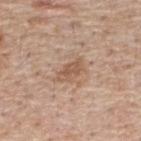Case summary:
– workup · catalogued during a skin exam; not biopsied
– patient · male, aged around 55
– image-analysis metrics · about 9 CIELAB-L* units darker than the surrounding skin and a lesion-to-skin contrast of about 6.5 (normalized; higher = more distinct); a border-irregularity index near 3.5/10 and a color-variation rating of about 2/10; a classifier nevus-likeness of about 45/100
– acquisition · 15 mm crop, total-body photography
– body site · the upper back
– diameter · about 3.5 mm